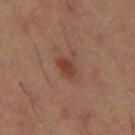Captured during whole-body skin photography for melanoma surveillance; the lesion was not biopsied.
From the leg.
The tile uses cross-polarized illumination.
Cropped from a whole-body photographic skin survey; the tile spans about 15 mm.
The recorded lesion diameter is about 3 mm.